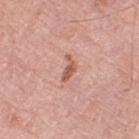<case>
<biopsy_status>not biopsied; imaged during a skin examination</biopsy_status>
<patient>
  <sex>male</sex>
  <age_approx>80</age_approx>
</patient>
<site>left thigh</site>
<automated_metrics>
  <area_mm2_approx>3.0</area_mm2_approx>
  <eccentricity>0.9</eccentricity>
  <shape_asymmetry>0.55</shape_asymmetry>
  <border_irregularity_0_10>5.5</border_irregularity_0_10>
  <color_variation_0_10>0.0</color_variation_0_10>
  <peripheral_color_asymmetry>0.0</peripheral_color_asymmetry>
</automated_metrics>
<image>
  <source>total-body photography crop</source>
  <field_of_view_mm>15</field_of_view_mm>
</image>
<lesion_size>
  <long_diameter_mm_approx>3.0</long_diameter_mm_approx>
</lesion_size>
</case>